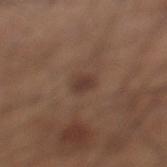Case summary:
– workup: total-body-photography surveillance lesion; no biopsy
– size: ~2.5 mm (longest diameter)
– site: the leg
– lighting: white-light
– acquisition: 15 mm crop, total-body photography
– image-analysis metrics: an area of roughly 3.5 mm² and two-axis asymmetry of about 0.2
– patient: male, aged 58–62